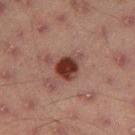{"biopsy_status": "not biopsied; imaged during a skin examination", "automated_metrics": {"area_mm2_approx": 6.5, "eccentricity": 0.35, "shape_asymmetry": 0.25, "lesion_detection_confidence_0_100": 100}, "site": "left thigh", "image": {"source": "total-body photography crop", "field_of_view_mm": 15}, "lighting": "cross-polarized", "lesion_size": {"long_diameter_mm_approx": 3.0}, "patient": {"sex": "male", "age_approx": 45}}